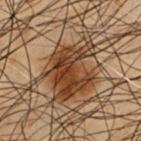Acquisition and patient details:
A region of skin cropped from a whole-body photographic capture, roughly 15 mm wide. Captured under cross-polarized illumination. The recorded lesion diameter is about 6 mm. From the front of the torso. Automated image analysis of the tile measured a lesion color around L≈32 a*≈18 b*≈28 in CIELAB, a lesion–skin lightness drop of about 11, and a normalized lesion–skin contrast near 10.5. The analysis additionally found border irregularity of about 6.5 on a 0–10 scale, a color-variation rating of about 5/10, and peripheral color asymmetry of about 2. The patient is a male approximately 55 years of age.